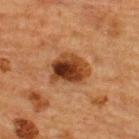Imaged during a routine full-body skin examination; the lesion was not biopsied and no histopathology is available.
Located on the upper back.
A male patient aged 63 to 67.
Captured under cross-polarized illumination.
A 15 mm close-up tile from a total-body photography series done for melanoma screening.
Automated tile analysis of the lesion measured an area of roughly 12 mm², a shape eccentricity near 0.65, and two-axis asymmetry of about 0.25. It also reported a mean CIELAB color near L≈34 a*≈22 b*≈32, roughly 15 lightness units darker than nearby skin, and a normalized border contrast of about 12. It also reported a color-variation rating of about 9.5/10 and a peripheral color-asymmetry measure near 3. The software also gave lesion-presence confidence of about 100/100.